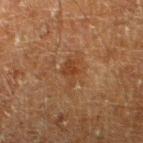<lesion>
  <biopsy_status>not biopsied; imaged during a skin examination</biopsy_status>
  <image>
    <source>total-body photography crop</source>
    <field_of_view_mm>15</field_of_view_mm>
  </image>
  <site>leg</site>
  <lesion_size>
    <long_diameter_mm_approx>3.0</long_diameter_mm_approx>
  </lesion_size>
  <lighting>cross-polarized</lighting>
  <patient>
    <sex>male</sex>
    <age_approx>60</age_approx>
  </patient>
  <automated_metrics>
    <lesion_detection_confidence_0_100>100</lesion_detection_confidence_0_100>
  </automated_metrics>
</lesion>